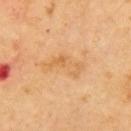Q: How was the tile lit?
A: cross-polarized illumination
Q: Where on the body is the lesion?
A: the arm
Q: What are the patient's age and sex?
A: male, aged around 70
Q: How large is the lesion?
A: ≈5 mm
Q: What kind of image is this?
A: 15 mm crop, total-body photography